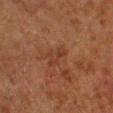This lesion was catalogued during total-body skin photography and was not selected for biopsy. Cropped from a whole-body photographic skin survey; the tile spans about 15 mm. The lesion's longest dimension is about 3 mm. The lesion is located on the left lower leg. Imaged with cross-polarized lighting. A male subject aged approximately 80.